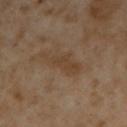• patient · male, approximately 55 years of age
• imaging modality · total-body-photography crop, ~15 mm field of view
• lesion diameter · ~5.5 mm (longest diameter)
• site · the arm
• illumination · cross-polarized